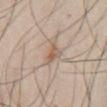| field | value |
|---|---|
| notes | no biopsy performed (imaged during a skin exam) |
| imaging modality | ~15 mm tile from a whole-body skin photo |
| location | the front of the torso |
| lighting | white-light illumination |
| subject | male, aged 43 to 47 |
| diameter | about 2.5 mm |